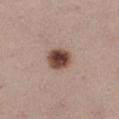Impression: Imaged during a routine full-body skin examination; the lesion was not biopsied and no histopathology is available. Background: A female subject aged around 25. A lesion tile, about 15 mm wide, cut from a 3D total-body photograph. The lesion-visualizer software estimated a footprint of about 7.5 mm² and an outline eccentricity of about 0.5 (0 = round, 1 = elongated). And it measured a lesion color around L≈45 a*≈19 b*≈24 in CIELAB, roughly 18 lightness units darker than nearby skin, and a normalized lesion–skin contrast near 13. The software also gave border irregularity of about 1.5 on a 0–10 scale, a color-variation rating of about 6/10, and radial color variation of about 2. Imaged with white-light lighting. The lesion is located on the left thigh.